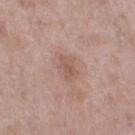Clinical impression: No biopsy was performed on this lesion — it was imaged during a full skin examination and was not determined to be concerning. Context: The patient is a female aged 58 to 62. The lesion is on the left thigh. A 15 mm close-up tile from a total-body photography series done for melanoma screening.